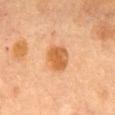  biopsy_status: not biopsied; imaged during a skin examination
  lesion_size:
    long_diameter_mm_approx: 3.0
  lighting: cross-polarized
  image:
    source: total-body photography crop
    field_of_view_mm: 15
  patient:
    sex: female
    age_approx: 60
  automated_metrics:
    cielab_L: 54
    cielab_a: 23
    cielab_b: 40
    vs_skin_darker_L: 11.0
    vs_skin_contrast_norm: 8.5
  site: chest